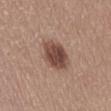<tbp_lesion>
  <lighting>white-light</lighting>
  <patient>
    <sex>female</sex>
    <age_approx>35</age_approx>
  </patient>
  <image>
    <source>total-body photography crop</source>
    <field_of_view_mm>15</field_of_view_mm>
  </image>
  <site>abdomen</site>
  <lesion_size>
    <long_diameter_mm_approx>4.0</long_diameter_mm_approx>
  </lesion_size>
  <automated_metrics>
    <area_mm2_approx>10.0</area_mm2_approx>
    <eccentricity>0.75</eccentricity>
    <shape_asymmetry>0.15</shape_asymmetry>
    <border_irregularity_0_10>1.5</border_irregularity_0_10>
    <color_variation_0_10>4.0</color_variation_0_10>
    <peripheral_color_asymmetry>1.5</peripheral_color_asymmetry>
  </automated_metrics>
</tbp_lesion>